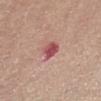The lesion was tiled from a total-body skin photograph and was not biopsied. This is a white-light tile. Automated tile analysis of the lesion measured a lesion color around L≈51 a*≈32 b*≈22 in CIELAB, roughly 14 lightness units darker than nearby skin, and a normalized lesion–skin contrast near 9.5. A female patient aged 48–52. A close-up tile cropped from a whole-body skin photograph, about 15 mm across. Located on the abdomen. The recorded lesion diameter is about 2.5 mm.